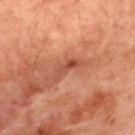No biopsy was performed on this lesion — it was imaged during a full skin examination and was not determined to be concerning. The total-body-photography lesion software estimated a nevus-likeness score of about 0/100. Cropped from a whole-body photographic skin survey; the tile spans about 15 mm. The lesion is located on the chest. This is a cross-polarized tile. A male patient aged around 65.